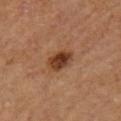subject = female, approximately 55 years of age
site = the left upper arm
lighting = cross-polarized illumination
automated lesion analysis = a nevus-likeness score of about 95/100
diameter = about 3 mm
imaging modality = total-body-photography crop, ~15 mm field of view